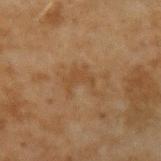• biopsy status — catalogued during a skin exam; not biopsied
• acquisition — ~15 mm tile from a whole-body skin photo
• patient — male, approximately 45 years of age
• location — the left upper arm
• lesion diameter — ~3.5 mm (longest diameter)
• tile lighting — cross-polarized